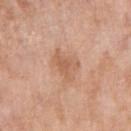Assessment: Part of a total-body skin-imaging series; this lesion was reviewed on a skin check and was not flagged for biopsy. Context: A roughly 15 mm field-of-view crop from a total-body skin photograph. A female patient, aged approximately 70. Longest diameter approximately 4 mm. Imaged with white-light lighting. From the right upper arm.